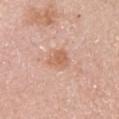notes: no biopsy performed (imaged during a skin exam)
imaging modality: 15 mm crop, total-body photography
patient: female, approximately 50 years of age
site: the left upper arm